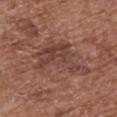Recorded during total-body skin imaging; not selected for excision or biopsy.
This is a white-light tile.
A female subject, aged around 75.
The lesion is on the chest.
A 15 mm close-up tile from a total-body photography series done for melanoma screening.
Approximately 7 mm at its widest.